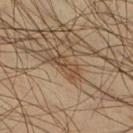Impression: Recorded during total-body skin imaging; not selected for excision or biopsy. Background: Longest diameter approximately 3.5 mm. A male patient, approximately 45 years of age. On the right lower leg. A region of skin cropped from a whole-body photographic capture, roughly 15 mm wide. Captured under cross-polarized illumination.